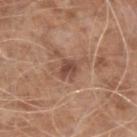notes — total-body-photography surveillance lesion; no biopsy
image source — ~15 mm crop, total-body skin-cancer survey
lesion diameter — about 2.5 mm
location — the right upper arm
subject — male, aged 58–62